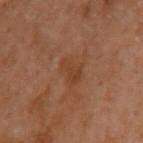The lesion was photographed on a routine skin check and not biopsied; there is no pathology result.
Longest diameter approximately 4 mm.
The lesion-visualizer software estimated a border-irregularity index near 4/10 and a within-lesion color-variation index near 1/10. The analysis additionally found lesion-presence confidence of about 100/100.
A region of skin cropped from a whole-body photographic capture, roughly 15 mm wide.
On the left arm.
The patient is a female approximately 60 years of age.
This is a cross-polarized tile.